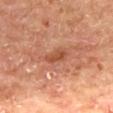Captured during whole-body skin photography for melanoma surveillance; the lesion was not biopsied. The lesion is located on the mid back. Imaged with cross-polarized lighting. Approximately 3.5 mm at its widest. A region of skin cropped from a whole-body photographic capture, roughly 15 mm wide. A male subject roughly 65 years of age. An algorithmic analysis of the crop reported a border-irregularity rating of about 3/10 and radial color variation of about 0.5.